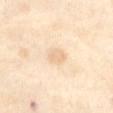biopsy status = no biopsy performed (imaged during a skin exam); subject = female, aged 53 to 57; image source = ~15 mm crop, total-body skin-cancer survey; size = ≈2.5 mm; body site = the abdomen; illumination = cross-polarized.